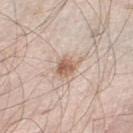Q: Is there a histopathology result?
A: total-body-photography surveillance lesion; no biopsy
Q: How was the tile lit?
A: white-light illumination
Q: What is the anatomic site?
A: the left lower leg
Q: What kind of image is this?
A: ~15 mm tile from a whole-body skin photo
Q: Who is the patient?
A: male, aged around 60